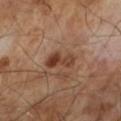Imaged during a routine full-body skin examination; the lesion was not biopsied and no histopathology is available. A 15 mm close-up extracted from a 3D total-body photography capture. The total-body-photography lesion software estimated an outline eccentricity of about 0.85 (0 = round, 1 = elongated) and a shape-asymmetry score of about 0.3 (0 = symmetric). The analysis additionally found internal color variation of about 7 on a 0–10 scale and radial color variation of about 3. The analysis additionally found a nevus-likeness score of about 55/100. This is a cross-polarized tile. About 3.5 mm across. A male subject aged 63 to 67.